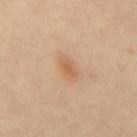Part of a total-body skin-imaging series; this lesion was reviewed on a skin check and was not flagged for biopsy. The total-body-photography lesion software estimated a footprint of about 3 mm², an eccentricity of roughly 0.85, and a symmetry-axis asymmetry near 0.35. The analysis additionally found a mean CIELAB color near L≈58 a*≈20 b*≈35, a lesion–skin lightness drop of about 8, and a normalized border contrast of about 6. On the mid back. Cropped from a whole-body photographic skin survey; the tile spans about 15 mm. A male subject aged 48 to 52. The lesion's longest dimension is about 2.5 mm.